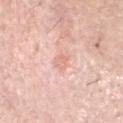| field | value |
|---|---|
| biopsy status | no biopsy performed (imaged during a skin exam) |
| patient | male, aged 58 to 62 |
| anatomic site | the head or neck |
| acquisition | ~15 mm crop, total-body skin-cancer survey |
| lesion size | about 2.5 mm |
| illumination | white-light illumination |